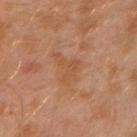Q: Was this lesion biopsied?
A: no biopsy performed (imaged during a skin exam)
Q: Illumination type?
A: cross-polarized
Q: Automated lesion metrics?
A: a classifier nevus-likeness of about 0/100
Q: What is the anatomic site?
A: the left arm
Q: Patient demographics?
A: male, aged around 30
Q: What is the lesion's diameter?
A: ~4.5 mm (longest diameter)
Q: How was this image acquired?
A: total-body-photography crop, ~15 mm field of view